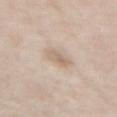The lesion was photographed on a routine skin check and not biopsied; there is no pathology result.
Located on the chest.
This image is a 15 mm lesion crop taken from a total-body photograph.
The subject is a male about 60 years old.
The lesion's longest dimension is about 3 mm.